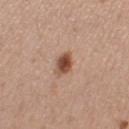Assessment:
This lesion was catalogued during total-body skin photography and was not selected for biopsy.
Acquisition and patient details:
The subject is a female about 30 years old. Cropped from a whole-body photographic skin survey; the tile spans about 15 mm. The tile uses white-light illumination. Automated tile analysis of the lesion measured a lesion color around L≈49 a*≈22 b*≈29 in CIELAB, about 15 CIELAB-L* units darker than the surrounding skin, and a normalized border contrast of about 10.5. Located on the leg. The lesion's longest dimension is about 3 mm.